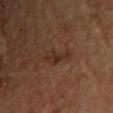biopsy_status: not biopsied; imaged during a skin examination
lighting: cross-polarized
automated_metrics:
  eccentricity: 0.85
  shape_asymmetry: 0.35
  border_irregularity_0_10: 4.0
  color_variation_0_10: 2.5
patient:
  sex: female
  age_approx: 60
lesion_size:
  long_diameter_mm_approx: 4.0
image:
  source: total-body photography crop
  field_of_view_mm: 15
site: chest